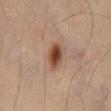Imaged during a routine full-body skin examination; the lesion was not biopsied and no histopathology is available. A male subject aged 53 to 57. A close-up tile cropped from a whole-body skin photograph, about 15 mm across. The tile uses cross-polarized illumination. About 3.5 mm across. On the abdomen.